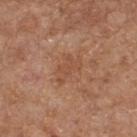notes = total-body-photography surveillance lesion; no biopsy | acquisition = 15 mm crop, total-body photography | site = the upper back | patient = male, aged around 65.